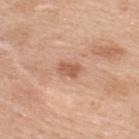Q: Was this lesion biopsied?
A: total-body-photography surveillance lesion; no biopsy
Q: What lighting was used for the tile?
A: white-light
Q: Who is the patient?
A: male, about 50 years old
Q: How large is the lesion?
A: ~2.5 mm (longest diameter)
Q: Lesion location?
A: the upper back
Q: What kind of image is this?
A: total-body-photography crop, ~15 mm field of view